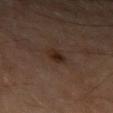Part of a total-body skin-imaging series; this lesion was reviewed on a skin check and was not flagged for biopsy. The total-body-photography lesion software estimated an outline eccentricity of about 0.55 (0 = round, 1 = elongated). And it measured a border-irregularity index near 2.5/10 and internal color variation of about 3 on a 0–10 scale. It also reported a nevus-likeness score of about 90/100 and a lesion-detection confidence of about 100/100. Located on the arm. A male subject aged 63–67. The tile uses cross-polarized illumination. The lesion's longest dimension is about 2 mm. A 15 mm crop from a total-body photograph taken for skin-cancer surveillance.